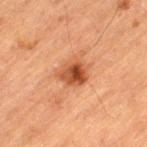biopsy status: imaged on a skin check; not biopsied | subject: male, about 85 years old or older | image: 15 mm crop, total-body photography | anatomic site: the left thigh.